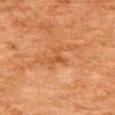This lesion was catalogued during total-body skin photography and was not selected for biopsy. The lesion is on the upper back. A male patient, in their 80s. A 15 mm close-up extracted from a 3D total-body photography capture. This is a cross-polarized tile. The recorded lesion diameter is about 3 mm.